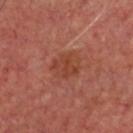{
  "biopsy_status": "not biopsied; imaged during a skin examination",
  "image": {
    "source": "total-body photography crop",
    "field_of_view_mm": 15
  },
  "patient": {
    "sex": "male",
    "age_approx": 60
  },
  "lesion_size": {
    "long_diameter_mm_approx": 3.5
  },
  "site": "head or neck",
  "automated_metrics": {
    "vs_skin_darker_L": 6.0,
    "vs_skin_contrast_norm": 6.0
  }
}